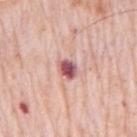workup: no biopsy performed (imaged during a skin exam) | acquisition: ~15 mm tile from a whole-body skin photo | anatomic site: the mid back | patient: male, roughly 80 years of age | tile lighting: white-light illumination.